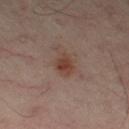The lesion was tiled from a total-body skin photograph and was not biopsied. From the left thigh. A male subject aged 48 to 52. Automated tile analysis of the lesion measured a footprint of about 5 mm², an outline eccentricity of about 0.7 (0 = round, 1 = elongated), and two-axis asymmetry of about 0.2. And it measured a within-lesion color-variation index near 3/10 and radial color variation of about 1. The analysis additionally found a classifier nevus-likeness of about 65/100 and a lesion-detection confidence of about 100/100. Measured at roughly 3 mm in maximum diameter. A 15 mm crop from a total-body photograph taken for skin-cancer surveillance.